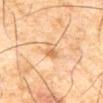Impression:
Imaged during a routine full-body skin examination; the lesion was not biopsied and no histopathology is available.
Image and clinical context:
A roughly 15 mm field-of-view crop from a total-body skin photograph. Imaged with cross-polarized lighting. The total-body-photography lesion software estimated an area of roughly 4 mm² and an outline eccentricity of about 0.8 (0 = round, 1 = elongated). The software also gave peripheral color asymmetry of about 1. The software also gave a classifier nevus-likeness of about 5/100 and a lesion-detection confidence of about 100/100. The lesion is on the abdomen. A male patient aged 63 to 67.